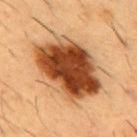Q: What are the patient's age and sex?
A: male, approximately 55 years of age
Q: What is the imaging modality?
A: 15 mm crop, total-body photography
Q: Where on the body is the lesion?
A: the chest